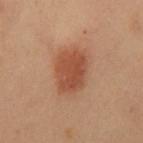Assessment: No biopsy was performed on this lesion — it was imaged during a full skin examination and was not determined to be concerning. Image and clinical context: A male subject aged 53–57. Imaged with cross-polarized lighting. From the front of the torso. The lesion's longest dimension is about 5 mm. Automated image analysis of the tile measured a mean CIELAB color near L≈39 a*≈21 b*≈27 and roughly 9 lightness units darker than nearby skin. The analysis additionally found border irregularity of about 2 on a 0–10 scale, a color-variation rating of about 2.5/10, and a peripheral color-asymmetry measure near 1. A region of skin cropped from a whole-body photographic capture, roughly 15 mm wide.